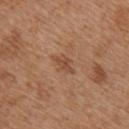{
  "biopsy_status": "not biopsied; imaged during a skin examination",
  "automated_metrics": {
    "vs_skin_darker_L": 8.0,
    "vs_skin_contrast_norm": 6.0
  },
  "site": "right upper arm",
  "lighting": "white-light",
  "patient": {
    "sex": "female",
    "age_approx": 40
  },
  "lesion_size": {
    "long_diameter_mm_approx": 3.0
  },
  "image": {
    "source": "total-body photography crop",
    "field_of_view_mm": 15
  }
}